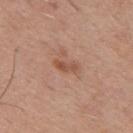follow-up: catalogued during a skin exam; not biopsied
image source: 15 mm crop, total-body photography
body site: the mid back
subject: male, aged around 30
image-analysis metrics: a border-irregularity rating of about 4/10, a color-variation rating of about 0/10, and peripheral color asymmetry of about 0
lesion size: about 3 mm
illumination: white-light illumination